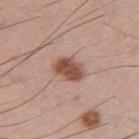The lesion was photographed on a routine skin check and not biopsied; there is no pathology result. This is a white-light tile. A roughly 15 mm field-of-view crop from a total-body skin photograph. Located on the right upper arm. A male subject approximately 35 years of age.